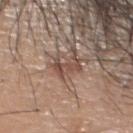Q: Was a biopsy performed?
A: imaged on a skin check; not biopsied
Q: What kind of image is this?
A: 15 mm crop, total-body photography
Q: Who is the patient?
A: male, approximately 45 years of age
Q: Lesion size?
A: ≈3 mm
Q: How was the tile lit?
A: white-light illumination
Q: What did automated image analysis measure?
A: an automated nevus-likeness rating near 0 out of 100 and a detector confidence of about 90 out of 100 that the crop contains a lesion
Q: Where on the body is the lesion?
A: the head or neck